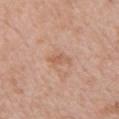Notes:
– workup — total-body-photography surveillance lesion; no biopsy
– imaging modality — total-body-photography crop, ~15 mm field of view
– tile lighting — white-light
– site — the chest
– lesion diameter — ≈2.5 mm
– TBP lesion metrics — a lesion area of about 2.5 mm² and two-axis asymmetry of about 0.45; a border-irregularity index near 5/10 and a within-lesion color-variation index near 0/10
– patient — male, in their mid-60s